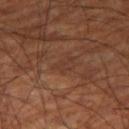{
  "biopsy_status": "not biopsied; imaged during a skin examination",
  "patient": {
    "sex": "male",
    "age_approx": 70
  },
  "automated_metrics": {
    "area_mm2_approx": 2.5,
    "eccentricity": 0.85,
    "shape_asymmetry": 0.55,
    "color_variation_0_10": 0.0,
    "peripheral_color_asymmetry": 0.0
  },
  "lesion_size": {
    "long_diameter_mm_approx": 2.5
  },
  "site": "right thigh",
  "lighting": "cross-polarized",
  "image": {
    "source": "total-body photography crop",
    "field_of_view_mm": 15
  }
}